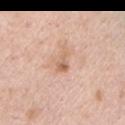<lesion>
  <biopsy_status>not biopsied; imaged during a skin examination</biopsy_status>
  <lesion_size>
    <long_diameter_mm_approx>3.0</long_diameter_mm_approx>
  </lesion_size>
  <site>left upper arm</site>
  <patient>
    <sex>male</sex>
    <age_approx>40</age_approx>
  </patient>
  <image>
    <source>total-body photography crop</source>
    <field_of_view_mm>15</field_of_view_mm>
  </image>
</lesion>